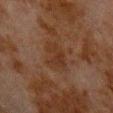Part of a total-body skin-imaging series; this lesion was reviewed on a skin check and was not flagged for biopsy. The total-body-photography lesion software estimated a lesion area of about 7.5 mm², an outline eccentricity of about 0.75 (0 = round, 1 = elongated), and a shape-asymmetry score of about 0.25 (0 = symmetric). And it measured a nevus-likeness score of about 0/100 and a lesion-detection confidence of about 100/100. Cropped from a whole-body photographic skin survey; the tile spans about 15 mm. A male subject aged approximately 80. On the chest.